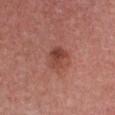The lesion was tiled from a total-body skin photograph and was not biopsied. From the chest. A female patient aged around 40. A close-up tile cropped from a whole-body skin photograph, about 15 mm across. The tile uses white-light illumination.